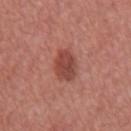Part of a total-body skin-imaging series; this lesion was reviewed on a skin check and was not flagged for biopsy. The lesion is located on the mid back. Cropped from a total-body skin-imaging series; the visible field is about 15 mm. The subject is a male aged around 65. The lesion-visualizer software estimated a footprint of about 8 mm², an eccentricity of roughly 0.6, and a symmetry-axis asymmetry near 0.15. The software also gave border irregularity of about 1.5 on a 0–10 scale and radial color variation of about 1.5. And it measured a classifier nevus-likeness of about 95/100 and lesion-presence confidence of about 100/100. Imaged with white-light lighting.